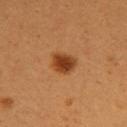  biopsy_status: not biopsied; imaged during a skin examination
  image:
    source: total-body photography crop
    field_of_view_mm: 15
  patient:
    sex: female
    age_approx: 25
  site: upper back
  lighting: cross-polarized
  automated_metrics:
    area_mm2_approx: 6.0
    eccentricity: 0.6
    shape_asymmetry: 0.2
    cielab_L: 40
    cielab_a: 26
    cielab_b: 38
  lesion_size:
    long_diameter_mm_approx: 3.0